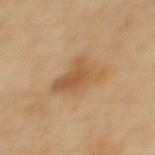anatomic site: the upper back
image source: ~15 mm tile from a whole-body skin photo
size: ~5.5 mm (longest diameter)
illumination: cross-polarized illumination
subject: female, approximately 70 years of age